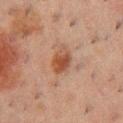| key | value |
|---|---|
| notes | no biopsy performed (imaged during a skin exam) |
| illumination | cross-polarized |
| diameter | ~3.5 mm (longest diameter) |
| image source | ~15 mm crop, total-body skin-cancer survey |
| subject | male, aged around 50 |
| automated lesion analysis | border irregularity of about 2 on a 0–10 scale, a color-variation rating of about 4.5/10, and radial color variation of about 1.5 |
| location | the chest |